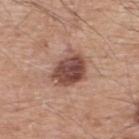Imaged during a routine full-body skin examination; the lesion was not biopsied and no histopathology is available. A male subject, in their mid-50s. On the upper back. A roughly 15 mm field-of-view crop from a total-body skin photograph.